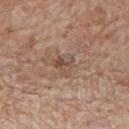notes: imaged on a skin check; not biopsied
tile lighting: white-light
anatomic site: the mid back
lesion diameter: ~2.5 mm (longest diameter)
patient: male, roughly 60 years of age
imaging modality: ~15 mm tile from a whole-body skin photo